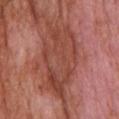Q: Is there a histopathology result?
A: no biopsy performed (imaged during a skin exam)
Q: How was the tile lit?
A: white-light illumination
Q: What is the imaging modality?
A: ~15 mm tile from a whole-body skin photo
Q: What is the anatomic site?
A: the back
Q: What did automated image analysis measure?
A: a mean CIELAB color near L≈45 a*≈27 b*≈29 and about 10 CIELAB-L* units darker than the surrounding skin; a border-irregularity rating of about 5.5/10, a color-variation rating of about 4.5/10, and radial color variation of about 1.5; an automated nevus-likeness rating near 0 out of 100 and a detector confidence of about 85 out of 100 that the crop contains a lesion
Q: What are the patient's age and sex?
A: male, in their mid-60s